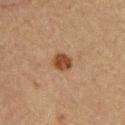This lesion was catalogued during total-body skin photography and was not selected for biopsy. Captured under cross-polarized illumination. A close-up tile cropped from a whole-body skin photograph, about 15 mm across. The lesion is on the front of the torso. A male patient roughly 40 years of age. About 2.5 mm across.